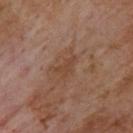Context:
Located on the upper back. The subject is a male aged approximately 70. Longest diameter approximately 3 mm. A lesion tile, about 15 mm wide, cut from a 3D total-body photograph. Captured under cross-polarized illumination.